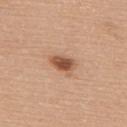Part of a total-body skin-imaging series; this lesion was reviewed on a skin check and was not flagged for biopsy. Automated tile analysis of the lesion measured a lesion area of about 5.5 mm² and a shape-asymmetry score of about 0.25 (0 = symmetric). It also reported border irregularity of about 2.5 on a 0–10 scale, a color-variation rating of about 3.5/10, and a peripheral color-asymmetry measure near 1. This is a white-light tile. A female subject aged around 65. A 15 mm close-up tile from a total-body photography series done for melanoma screening. On the upper back. About 3 mm across.